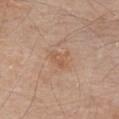This lesion was catalogued during total-body skin photography and was not selected for biopsy.
The lesion is on the front of the torso.
This is a white-light tile.
This image is a 15 mm lesion crop taken from a total-body photograph.
The patient is a male roughly 80 years of age.
About 3 mm across.